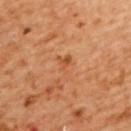notes: catalogued during a skin exam; not biopsied | image source: ~15 mm crop, total-body skin-cancer survey | site: the upper back | size: about 3 mm | subject: female, approximately 55 years of age | illumination: cross-polarized.